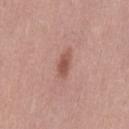biopsy_status: not biopsied; imaged during a skin examination
site: lower back
image:
  source: total-body photography crop
  field_of_view_mm: 15
patient:
  sex: female
  age_approx: 40
lesion_size:
  long_diameter_mm_approx: 3.0
automated_metrics:
  area_mm2_approx: 4.5
  eccentricity: 0.85
  shape_asymmetry: 0.2
  vs_skin_darker_L: 10.0
lighting: white-light